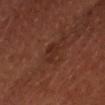biopsy status: no biopsy performed (imaged during a skin exam); size: about 3.5 mm; acquisition: ~15 mm crop, total-body skin-cancer survey; subject: male, aged around 45; anatomic site: the head or neck.